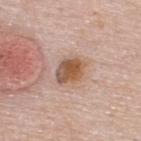Clinical impression: The lesion was tiled from a total-body skin photograph and was not biopsied.